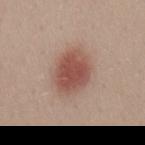Case summary:
• notes · catalogued during a skin exam; not biopsied
• patient · male, aged 28–32
• lighting · white-light illumination
• image · 15 mm crop, total-body photography
• diameter · ~5.5 mm (longest diameter)
• anatomic site · the back